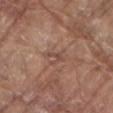Q: Patient demographics?
A: male, aged around 80
Q: Lesion location?
A: the mid back
Q: What is the lesion's diameter?
A: ≈2.5 mm
Q: Illumination type?
A: white-light illumination
Q: What kind of image is this?
A: total-body-photography crop, ~15 mm field of view